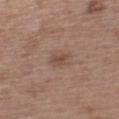{"biopsy_status": "not biopsied; imaged during a skin examination", "lighting": "white-light", "patient": {"sex": "male", "age_approx": 55}, "image": {"source": "total-body photography crop", "field_of_view_mm": 15}, "lesion_size": {"long_diameter_mm_approx": 2.5}, "automated_metrics": {"cielab_L": 48, "cielab_a": 18, "cielab_b": 25, "vs_skin_contrast_norm": 5.5}, "site": "left upper arm"}